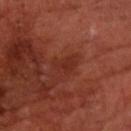{
  "lighting": "cross-polarized",
  "lesion_size": {
    "long_diameter_mm_approx": 3.0
  },
  "site": "front of the torso",
  "automated_metrics": {
    "vs_skin_darker_L": 5.0,
    "border_irregularity_0_10": 3.5,
    "color_variation_0_10": 1.5,
    "nevus_likeness_0_100": 0,
    "lesion_detection_confidence_0_100": 100
  },
  "image": {
    "source": "total-body photography crop",
    "field_of_view_mm": 15
  },
  "patient": {
    "sex": "male",
    "age_approx": 70
  }
}